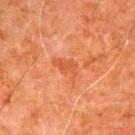<record>
<biopsy_status>not biopsied; imaged during a skin examination</biopsy_status>
<patient>
  <sex>male</sex>
  <age_approx>80</age_approx>
</patient>
<automated_metrics>
  <area_mm2_approx>5.0</area_mm2_approx>
  <eccentricity>0.8</eccentricity>
  <nevus_likeness_0_100>0</nevus_likeness_0_100>
</automated_metrics>
<site>arm</site>
<lighting>cross-polarized</lighting>
<lesion_size>
  <long_diameter_mm_approx>3.5</long_diameter_mm_approx>
</lesion_size>
<image>
  <source>total-body photography crop</source>
  <field_of_view_mm>15</field_of_view_mm>
</image>
</record>